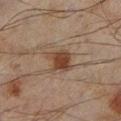This lesion was catalogued during total-body skin photography and was not selected for biopsy. Cropped from a whole-body photographic skin survey; the tile spans about 15 mm. From the left lower leg. The total-body-photography lesion software estimated a lesion color around L≈33 a*≈15 b*≈24 in CIELAB and a normalized lesion–skin contrast near 9.5. The software also gave a border-irregularity rating of about 2.5/10, internal color variation of about 3 on a 0–10 scale, and radial color variation of about 1. The software also gave an automated nevus-likeness rating near 95 out of 100 and a lesion-detection confidence of about 100/100. This is a cross-polarized tile. The patient is a male roughly 45 years of age. Longest diameter approximately 3 mm.